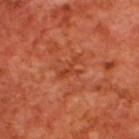Recorded during total-body skin imaging; not selected for excision or biopsy.
On the upper back.
Captured under cross-polarized illumination.
The lesion's longest dimension is about 3 mm.
A close-up tile cropped from a whole-body skin photograph, about 15 mm across.
A male subject aged 68 to 72.